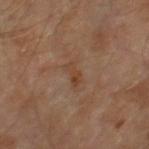No biopsy was performed on this lesion — it was imaged during a full skin examination and was not determined to be concerning. A region of skin cropped from a whole-body photographic capture, roughly 15 mm wide. The subject is a male aged around 60. The lesion is on the right leg. Imaged with cross-polarized lighting.